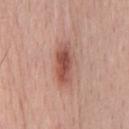follow-up: no biopsy performed (imaged during a skin exam)
image: ~15 mm crop, total-body skin-cancer survey
lesion size: ~5 mm (longest diameter)
tile lighting: white-light
subject: male, in their mid-50s
body site: the chest